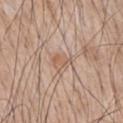Assessment:
The lesion was tiled from a total-body skin photograph and was not biopsied.
Clinical summary:
The lesion is located on the chest. Cropped from a whole-body photographic skin survey; the tile spans about 15 mm. The tile uses white-light illumination. Approximately 2.5 mm at its widest. A male subject aged 63–67.